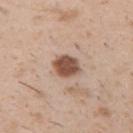Impression:
The lesion was tiled from a total-body skin photograph and was not biopsied.
Acquisition and patient details:
A region of skin cropped from a whole-body photographic capture, roughly 15 mm wide. Automated image analysis of the tile measured a border-irregularity index near 2/10, a color-variation rating of about 2.5/10, and radial color variation of about 1. A male patient, aged around 50. Approximately 3 mm at its widest. The tile uses white-light illumination. The lesion is located on the left upper arm.